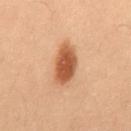No biopsy was performed on this lesion — it was imaged during a full skin examination and was not determined to be concerning. A region of skin cropped from a whole-body photographic capture, roughly 15 mm wide. From the mid back. Imaged with cross-polarized lighting. The patient is a male aged around 55.